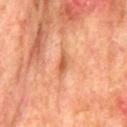{
  "patient": {
    "sex": "male",
    "age_approx": 75
  },
  "site": "mid back",
  "automated_metrics": {
    "color_variation_0_10": 0.0
  },
  "image": {
    "source": "total-body photography crop",
    "field_of_view_mm": 15
  }
}